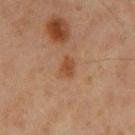Q: Was a biopsy performed?
A: total-body-photography surveillance lesion; no biopsy
Q: What kind of image is this?
A: ~15 mm crop, total-body skin-cancer survey
Q: Where on the body is the lesion?
A: the chest
Q: Lesion size?
A: about 2.5 mm
Q: Illumination type?
A: cross-polarized illumination
Q: Who is the patient?
A: male, approximately 60 years of age